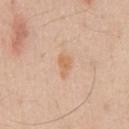Q: Was this lesion biopsied?
A: no biopsy performed (imaged during a skin exam)